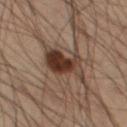{
  "biopsy_status": "not biopsied; imaged during a skin examination",
  "patient": {
    "sex": "male",
    "age_approx": 55
  },
  "image": {
    "source": "total-body photography crop",
    "field_of_view_mm": 15
  },
  "site": "leg"
}